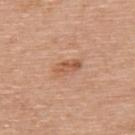Part of a total-body skin-imaging series; this lesion was reviewed on a skin check and was not flagged for biopsy. An algorithmic analysis of the crop reported a lesion area of about 5 mm², an eccentricity of roughly 0.9, and a shape-asymmetry score of about 0.2 (0 = symmetric). The software also gave a border-irregularity index near 2.5/10, internal color variation of about 4.5 on a 0–10 scale, and radial color variation of about 1.5. About 3.5 mm across. Imaged with white-light lighting. A male subject, aged approximately 80. On the upper back. This image is a 15 mm lesion crop taken from a total-body photograph.